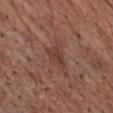| feature | finding |
|---|---|
| workup | catalogued during a skin exam; not biopsied |
| illumination | white-light illumination |
| subject | male, approximately 70 years of age |
| body site | the chest |
| image | 15 mm crop, total-body photography |